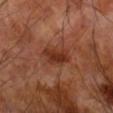Notes:
– workup: total-body-photography surveillance lesion; no biopsy
– subject: male, roughly 70 years of age
– tile lighting: cross-polarized
– acquisition: 15 mm crop, total-body photography
– anatomic site: the right forearm
– diameter: ~4 mm (longest diameter)